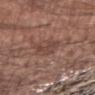* biopsy status — total-body-photography surveillance lesion; no biopsy
* patient — male, approximately 55 years of age
* TBP lesion metrics — a border-irregularity index near 6/10, a within-lesion color-variation index near 1/10, and radial color variation of about 0.5; an automated nevus-likeness rating near 0 out of 100 and lesion-presence confidence of about 95/100
* lighting — white-light
* lesion size — ≈3.5 mm
* imaging modality — ~15 mm tile from a whole-body skin photo
* body site — the right forearm